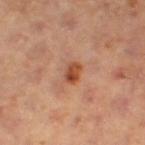Clinical impression:
The lesion was tiled from a total-body skin photograph and was not biopsied.
Image and clinical context:
The patient is a female aged around 40. A region of skin cropped from a whole-body photographic capture, roughly 15 mm wide. About 2.5 mm across. The lesion is on the right thigh. Captured under cross-polarized illumination.